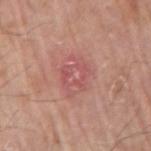Assessment: The lesion was tiled from a total-body skin photograph and was not biopsied. Image and clinical context: A 15 mm close-up extracted from a 3D total-body photography capture. Longest diameter approximately 4.5 mm. The lesion-visualizer software estimated a footprint of about 11 mm², an outline eccentricity of about 0.65 (0 = round, 1 = elongated), and two-axis asymmetry of about 0.2. The analysis additionally found a lesion color around L≈55 a*≈27 b*≈24 in CIELAB, a lesion–skin lightness drop of about 6, and a lesion-to-skin contrast of about 5 (normalized; higher = more distinct). And it measured a color-variation rating of about 4.5/10 and radial color variation of about 1.5. The tile uses white-light illumination. The lesion is located on the arm. A male patient aged around 70.